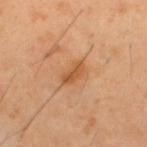Case summary:
– biopsy status — no biopsy performed (imaged during a skin exam)
– image source — ~15 mm crop, total-body skin-cancer survey
– location — the upper back
– automated lesion analysis — a mean CIELAB color near L≈54 a*≈23 b*≈39 and about 9 CIELAB-L* units darker than the surrounding skin
– subject — male, aged 38 to 42